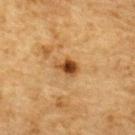Cropped from a total-body skin-imaging series; the visible field is about 15 mm. On the upper back. A male subject in their mid-80s or older.